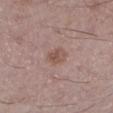biopsy_status: not biopsied; imaged during a skin examination
lesion_size:
  long_diameter_mm_approx: 2.5
lighting: white-light
image:
  source: total-body photography crop
  field_of_view_mm: 15
patient:
  sex: male
  age_approx: 50
site: leg